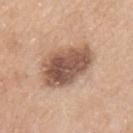Findings:
- workup: catalogued during a skin exam; not biopsied
- tile lighting: white-light illumination
- diameter: ≈6.5 mm
- image source: 15 mm crop, total-body photography
- patient: female, approximately 55 years of age
- image-analysis metrics: border irregularity of about 2 on a 0–10 scale; lesion-presence confidence of about 100/100
- site: the left upper arm